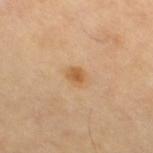Impression: This lesion was catalogued during total-body skin photography and was not selected for biopsy. Context: A female patient, roughly 70 years of age. This image is a 15 mm lesion crop taken from a total-body photograph. On the right thigh. The lesion's longest dimension is about 2 mm.